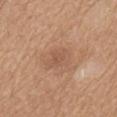The lesion was photographed on a routine skin check and not biopsied; there is no pathology result.